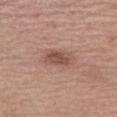The lesion was photographed on a routine skin check and not biopsied; there is no pathology result. The lesion is located on the left thigh. This is a white-light tile. A lesion tile, about 15 mm wide, cut from a 3D total-body photograph. The lesion-visualizer software estimated internal color variation of about 3.5 on a 0–10 scale and a peripheral color-asymmetry measure near 1. The analysis additionally found a nevus-likeness score of about 70/100 and lesion-presence confidence of about 100/100. The subject is a female approximately 65 years of age. The lesion's longest dimension is about 3.5 mm.